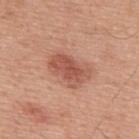site: upper back
image:
  source: total-body photography crop
  field_of_view_mm: 15
automated_metrics:
  border_irregularity_0_10: 2.5
  color_variation_0_10: 4.0
  peripheral_color_asymmetry: 1.5
patient:
  sex: male
  age_approx: 50
lighting: white-light
lesion_size:
  long_diameter_mm_approx: 5.0